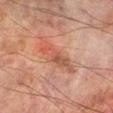biopsy status: catalogued during a skin exam; not biopsied
lesion diameter: ≈6.5 mm
automated lesion analysis: border irregularity of about 4.5 on a 0–10 scale and a color-variation rating of about 7.5/10
illumination: cross-polarized
location: the right lower leg
patient: male, aged approximately 70
image: total-body-photography crop, ~15 mm field of view